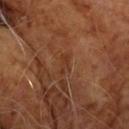{"biopsy_status": "not biopsied; imaged during a skin examination", "patient": {"sex": "male", "age_approx": 60}, "automated_metrics": {"eccentricity": 0.95, "shape_asymmetry": 0.55}, "site": "chest", "lighting": "cross-polarized", "image": {"source": "total-body photography crop", "field_of_view_mm": 15}}